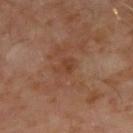Assessment:
The lesion was tiled from a total-body skin photograph and was not biopsied.